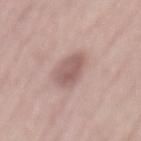notes — imaged on a skin check; not biopsied | image source — 15 mm crop, total-body photography | subject — male, aged 58–62 | location — the back.